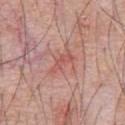<record>
  <biopsy_status>not biopsied; imaged during a skin examination</biopsy_status>
  <patient>
    <sex>male</sex>
    <age_approx>60</age_approx>
  </patient>
  <lesion_size>
    <long_diameter_mm_approx>3.5</long_diameter_mm_approx>
  </lesion_size>
  <image>
    <source>total-body photography crop</source>
    <field_of_view_mm>15</field_of_view_mm>
  </image>
  <automated_metrics>
    <area_mm2_approx>3.5</area_mm2_approx>
    <eccentricity>0.95</eccentricity>
    <shape_asymmetry>0.4</shape_asymmetry>
    <border_irregularity_0_10>4.5</border_irregularity_0_10>
    <color_variation_0_10>0.0</color_variation_0_10>
    <peripheral_color_asymmetry>0.0</peripheral_color_asymmetry>
    <nevus_likeness_0_100>0</nevus_likeness_0_100>
    <lesion_detection_confidence_0_100>95</lesion_detection_confidence_0_100>
  </automated_metrics>
  <site>chest</site>
  <lighting>white-light</lighting>
</record>